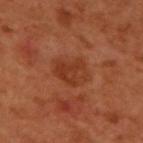{
  "biopsy_status": "not biopsied; imaged during a skin examination",
  "lighting": "cross-polarized",
  "patient": {
    "sex": "male",
    "age_approx": 50
  },
  "lesion_size": {
    "long_diameter_mm_approx": 4.5
  },
  "image": {
    "source": "total-body photography crop",
    "field_of_view_mm": 15
  },
  "site": "upper back"
}